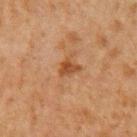| feature | finding |
|---|---|
| notes | catalogued during a skin exam; not biopsied |
| image | 15 mm crop, total-body photography |
| site | the left upper arm |
| patient | male, aged 58–62 |
| TBP lesion metrics | a border-irregularity index near 3/10, a color-variation rating of about 2.5/10, and peripheral color asymmetry of about 0.5; an automated nevus-likeness rating near 65 out of 100 and lesion-presence confidence of about 100/100 |
| lighting | cross-polarized |